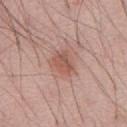biopsy_status: not biopsied; imaged during a skin examination
lesion_size:
  long_diameter_mm_approx: 2.5
automated_metrics:
  vs_skin_darker_L: 9.0
  vs_skin_contrast_norm: 6.5
lighting: white-light
image:
  source: total-body photography crop
  field_of_view_mm: 15
site: front of the torso
patient:
  sex: male
  age_approx: 55